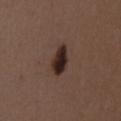biopsy status=imaged on a skin check; not biopsied | image=15 mm crop, total-body photography | tile lighting=white-light | image-analysis metrics=an eccentricity of roughly 0.85 and two-axis asymmetry of about 0.25; a mean CIELAB color near L≈25 a*≈16 b*≈19, roughly 15 lightness units darker than nearby skin, and a normalized border contrast of about 15; a within-lesion color-variation index near 4/10 and peripheral color asymmetry of about 1 | patient=female, approximately 50 years of age | location=the front of the torso.